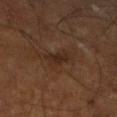Captured during whole-body skin photography for melanoma surveillance; the lesion was not biopsied. Imaged with cross-polarized lighting. A close-up tile cropped from a whole-body skin photograph, about 15 mm across. The lesion's longest dimension is about 3 mm. A male subject, aged approximately 65. The lesion is on the left lower leg.